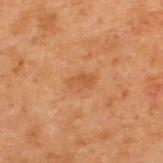follow-up = total-body-photography surveillance lesion; no biopsy
lesion diameter = ≈3 mm
acquisition = total-body-photography crop, ~15 mm field of view
patient = male, aged 68–72
location = the upper back
illumination = cross-polarized illumination
automated metrics = a footprint of about 4 mm², an eccentricity of roughly 0.85, and two-axis asymmetry of about 0.3; roughly 6 lightness units darker than nearby skin and a normalized border contrast of about 5